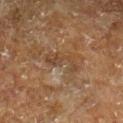Notes:
• workup: imaged on a skin check; not biopsied
• automated metrics: a mean CIELAB color near L≈32 a*≈14 b*≈25 and about 5 CIELAB-L* units darker than the surrounding skin; a nevus-likeness score of about 0/100
• patient: male, in their 60s
• image: 15 mm crop, total-body photography
• site: the right lower leg